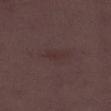This lesion was catalogued during total-body skin photography and was not selected for biopsy. On the left thigh. The patient is a female approximately 30 years of age. The lesion's longest dimension is about 3 mm. This image is a 15 mm lesion crop taken from a total-body photograph.